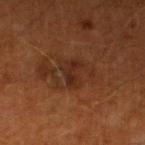Captured during whole-body skin photography for melanoma surveillance; the lesion was not biopsied. From the leg. A lesion tile, about 15 mm wide, cut from a 3D total-body photograph. The lesion's longest dimension is about 3.5 mm. A male patient, about 60 years old.